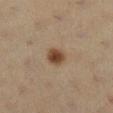• follow-up · no biopsy performed (imaged during a skin exam)
• anatomic site · the leg
• patient · female, aged approximately 40
• image · total-body-photography crop, ~15 mm field of view